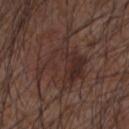Image and clinical context:
A 15 mm crop from a total-body photograph taken for skin-cancer surveillance. Captured under white-light illumination. The patient is a male aged 48 to 52. The lesion-visualizer software estimated a nevus-likeness score of about 20/100. The lesion is on the arm.